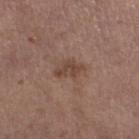Impression:
Imaged during a routine full-body skin examination; the lesion was not biopsied and no histopathology is available.
Background:
A region of skin cropped from a whole-body photographic capture, roughly 15 mm wide. A female subject, approximately 65 years of age. The lesion is on the left lower leg.